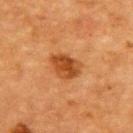Imaged during a routine full-body skin examination; the lesion was not biopsied and no histopathology is available.